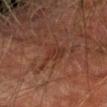Recorded during total-body skin imaging; not selected for excision or biopsy.
This is a cross-polarized tile.
The subject is a male aged 73 to 77.
The recorded lesion diameter is about 4.5 mm.
A close-up tile cropped from a whole-body skin photograph, about 15 mm across.
The lesion is located on the left forearm.
The lesion-visualizer software estimated a border-irregularity rating of about 6.5/10, internal color variation of about 2.5 on a 0–10 scale, and a peripheral color-asymmetry measure near 1. And it measured a nevus-likeness score of about 0/100 and a detector confidence of about 100 out of 100 that the crop contains a lesion.